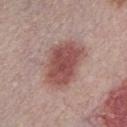notes = catalogued during a skin exam; not biopsied | patient = male, aged around 30 | imaging modality = ~15 mm tile from a whole-body skin photo.